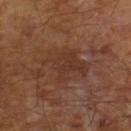Case summary:
- follow-up: catalogued during a skin exam; not biopsied
- subject: male, aged 63 to 67
- size: ~5 mm (longest diameter)
- image: ~15 mm crop, total-body skin-cancer survey
- TBP lesion metrics: a lesion area of about 16 mm² and an outline eccentricity of about 0.45 (0 = round, 1 = elongated); an average lesion color of about L≈34 a*≈19 b*≈26 (CIELAB), roughly 5 lightness units darker than nearby skin, and a normalized lesion–skin contrast near 5